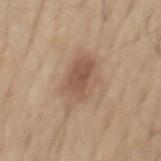Impression:
The lesion was tiled from a total-body skin photograph and was not biopsied.
Context:
Captured under white-light illumination. A region of skin cropped from a whole-body photographic capture, roughly 15 mm wide. Measured at roughly 5.5 mm in maximum diameter. On the mid back. Automated image analysis of the tile measured an average lesion color of about L≈54 a*≈17 b*≈28 (CIELAB), about 10 CIELAB-L* units darker than the surrounding skin, and a lesion-to-skin contrast of about 7 (normalized; higher = more distinct). And it measured a lesion-detection confidence of about 100/100. A male subject roughly 65 years of age.